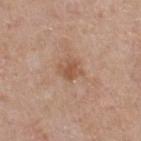{
  "biopsy_status": "not biopsied; imaged during a skin examination",
  "image": {
    "source": "total-body photography crop",
    "field_of_view_mm": 15
  },
  "patient": {
    "sex": "male",
    "age_approx": 55
  }
}